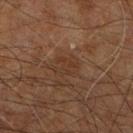Q: Was this lesion biopsied?
A: imaged on a skin check; not biopsied
Q: What is the imaging modality?
A: ~15 mm tile from a whole-body skin photo
Q: What are the patient's age and sex?
A: male, about 60 years old
Q: Lesion location?
A: the right leg
Q: What did automated image analysis measure?
A: an eccentricity of roughly 0.65 and two-axis asymmetry of about 0.3; a lesion–skin lightness drop of about 5 and a normalized lesion–skin contrast near 4.5
Q: What lighting was used for the tile?
A: cross-polarized